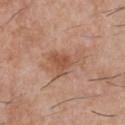notes: imaged on a skin check; not biopsied
body site: the chest
patient: male, aged around 30
lighting: white-light
image: ~15 mm crop, total-body skin-cancer survey
lesion diameter: ~5.5 mm (longest diameter)
automated metrics: a lesion area of about 12 mm² and a shape-asymmetry score of about 0.4 (0 = symmetric); border irregularity of about 4.5 on a 0–10 scale and a peripheral color-asymmetry measure near 1; a nevus-likeness score of about 40/100 and a detector confidence of about 100 out of 100 that the crop contains a lesion